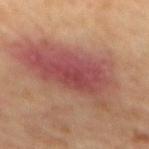<case>
<biopsy_status>not biopsied; imaged during a skin examination</biopsy_status>
<automated_metrics>
  <area_mm2_approx>50.0</area_mm2_approx>
  <shape_asymmetry>0.3</shape_asymmetry>
  <border_irregularity_0_10>6.0</border_irregularity_0_10>
  <peripheral_color_asymmetry>2.5</peripheral_color_asymmetry>
</automated_metrics>
<site>mid back</site>
<lighting>cross-polarized</lighting>
<image>
  <source>total-body photography crop</source>
  <field_of_view_mm>15</field_of_view_mm>
</image>
<patient>
  <sex>female</sex>
  <age_approx>60</age_approx>
</patient>
<lesion_size>
  <long_diameter_mm_approx>13.5</long_diameter_mm_approx>
</lesion_size>
</case>